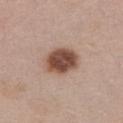Clinical impression: Imaged during a routine full-body skin examination; the lesion was not biopsied and no histopathology is available. Acquisition and patient details: The recorded lesion diameter is about 4 mm. The patient is a female roughly 40 years of age. The tile uses white-light illumination. A 15 mm crop from a total-body photograph taken for skin-cancer surveillance. The lesion is located on the abdomen.